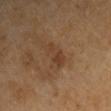On the left arm. Imaged with cross-polarized lighting. A lesion tile, about 15 mm wide, cut from a 3D total-body photograph. The recorded lesion diameter is about 4 mm. A male subject, about 65 years old. Automated tile analysis of the lesion measured an outline eccentricity of about 0.95 (0 = round, 1 = elongated) and a shape-asymmetry score of about 0.45 (0 = symmetric). The software also gave a border-irregularity rating of about 5.5/10, a color-variation rating of about 1/10, and peripheral color asymmetry of about 0. The software also gave an automated nevus-likeness rating near 0 out of 100 and a lesion-detection confidence of about 100/100.